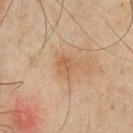Q: Was a biopsy performed?
A: no biopsy performed (imaged during a skin exam)
Q: Illumination type?
A: cross-polarized illumination
Q: Who is the patient?
A: male, aged approximately 45
Q: Where on the body is the lesion?
A: the chest
Q: How was this image acquired?
A: total-body-photography crop, ~15 mm field of view
Q: How large is the lesion?
A: ≈2.5 mm